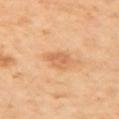Captured during whole-body skin photography for melanoma surveillance; the lesion was not biopsied. The subject is a female aged around 45. Cropped from a total-body skin-imaging series; the visible field is about 15 mm. Located on the left upper arm.